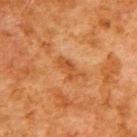| key | value |
|---|---|
| notes | total-body-photography surveillance lesion; no biopsy |
| subject | male, about 80 years old |
| lighting | cross-polarized illumination |
| lesion diameter | ≈3 mm |
| anatomic site | the back |
| image source | total-body-photography crop, ~15 mm field of view |
| automated lesion analysis | a mean CIELAB color near L≈40 a*≈23 b*≈36 and a lesion–skin lightness drop of about 7; a detector confidence of about 100 out of 100 that the crop contains a lesion |